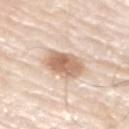workup — catalogued during a skin exam; not biopsied | lesion size — ≈4 mm | TBP lesion metrics — a mean CIELAB color near L≈66 a*≈18 b*≈30 and a lesion-to-skin contrast of about 8.5 (normalized; higher = more distinct); an automated nevus-likeness rating near 95 out of 100 | illumination — white-light illumination | location — the arm | subject — male, aged around 80 | imaging modality — ~15 mm crop, total-body skin-cancer survey.